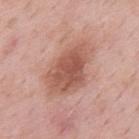Q: Is there a histopathology result?
A: no biopsy performed (imaged during a skin exam)
Q: How was this image acquired?
A: ~15 mm crop, total-body skin-cancer survey
Q: How was the tile lit?
A: white-light illumination
Q: What are the patient's age and sex?
A: male, roughly 55 years of age
Q: What is the anatomic site?
A: the mid back